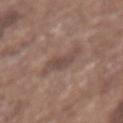| key | value |
|---|---|
| biopsy status | imaged on a skin check; not biopsied |
| subject | female, aged 73 to 77 |
| location | the chest |
| size | ~3.5 mm (longest diameter) |
| automated metrics | an area of roughly 6.5 mm² and a symmetry-axis asymmetry near 0.4; a mean CIELAB color near L≈47 a*≈17 b*≈22, about 8 CIELAB-L* units darker than the surrounding skin, and a normalized border contrast of about 6; a peripheral color-asymmetry measure near 0.5; a nevus-likeness score of about 0/100 and lesion-presence confidence of about 100/100 |
| image source | ~15 mm tile from a whole-body skin photo |
| tile lighting | white-light |